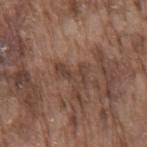{"image": {"source": "total-body photography crop", "field_of_view_mm": 15}, "lighting": "white-light", "patient": {"sex": "male", "age_approx": 75}, "lesion_size": {"long_diameter_mm_approx": 4.0}, "site": "back"}